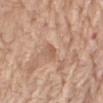Q: Who is the patient?
A: female, aged 73 to 77
Q: How was this image acquired?
A: ~15 mm crop, total-body skin-cancer survey
Q: How was the tile lit?
A: white-light
Q: Where on the body is the lesion?
A: the right thigh